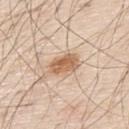No biopsy was performed on this lesion — it was imaged during a full skin examination and was not determined to be concerning.
About 4 mm across.
An algorithmic analysis of the crop reported a footprint of about 8 mm², a shape eccentricity near 0.75, and a symmetry-axis asymmetry near 0.15. And it measured a detector confidence of about 100 out of 100 that the crop contains a lesion.
A male patient aged 78–82.
A 15 mm close-up extracted from a 3D total-body photography capture.
Located on the back.